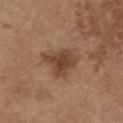<case>
<biopsy_status>not biopsied; imaged during a skin examination</biopsy_status>
<patient>
  <sex>female</sex>
  <age_approx>65</age_approx>
</patient>
<lesion_size>
  <long_diameter_mm_approx>5.0</long_diameter_mm_approx>
</lesion_size>
<site>front of the torso</site>
<image>
  <source>total-body photography crop</source>
  <field_of_view_mm>15</field_of_view_mm>
</image>
<automated_metrics>
  <area_mm2_approx>12.0</area_mm2_approx>
  <shape_asymmetry>0.35</shape_asymmetry>
  <border_irregularity_0_10>4.0</border_irregularity_0_10>
  <color_variation_0_10>3.5</color_variation_0_10>
  <peripheral_color_asymmetry>1.0</peripheral_color_asymmetry>
</automated_metrics>
<lighting>white-light</lighting>
</case>